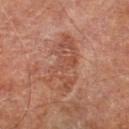The lesion was photographed on a routine skin check and not biopsied; there is no pathology result. The patient is a male aged approximately 70. Located on the left lower leg. Automated image analysis of the tile measured an area of roughly 17 mm², a shape eccentricity near 0.9, and a shape-asymmetry score of about 0.3 (0 = symmetric). It also reported border irregularity of about 6 on a 0–10 scale, a within-lesion color-variation index near 4/10, and radial color variation of about 1. It also reported a lesion-detection confidence of about 100/100. The lesion's longest dimension is about 7 mm. This image is a 15 mm lesion crop taken from a total-body photograph. The tile uses cross-polarized illumination.